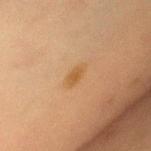The lesion was tiled from a total-body skin photograph and was not biopsied. A 15 mm crop from a total-body photograph taken for skin-cancer surveillance. A female patient about 55 years old. On the chest. The recorded lesion diameter is about 3 mm. This is a cross-polarized tile.